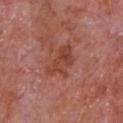{"biopsy_status": "not biopsied; imaged during a skin examination", "lesion_size": {"long_diameter_mm_approx": 4.5}, "patient": {"sex": "male", "age_approx": 65}, "image": {"source": "total-body photography crop", "field_of_view_mm": 15}, "site": "chest", "lighting": "white-light"}